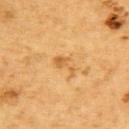Recorded during total-body skin imaging; not selected for excision or biopsy. About 3.5 mm across. Cropped from a whole-body photographic skin survey; the tile spans about 15 mm. A male patient, aged 83 or older. On the upper back. This is a cross-polarized tile.